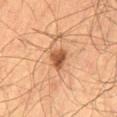Clinical impression:
Recorded during total-body skin imaging; not selected for excision or biopsy.
Acquisition and patient details:
A roughly 15 mm field-of-view crop from a total-body skin photograph. Located on the left thigh. The patient is a male approximately 65 years of age.